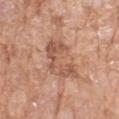No biopsy was performed on this lesion — it was imaged during a full skin examination and was not determined to be concerning. The subject is a female approximately 75 years of age. Cropped from a whole-body photographic skin survey; the tile spans about 15 mm. Measured at roughly 5.5 mm in maximum diameter. This is a white-light tile. Located on the left forearm.